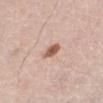notes — imaged on a skin check; not biopsied | tile lighting — white-light | image source — ~15 mm crop, total-body skin-cancer survey | site — the abdomen | lesion diameter — ~2.5 mm (longest diameter) | patient — female, aged approximately 65 | automated lesion analysis — a footprint of about 4 mm², an outline eccentricity of about 0.7 (0 = round, 1 = elongated), and a symmetry-axis asymmetry near 0.2.